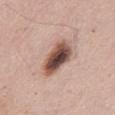Clinical impression: This lesion was catalogued during total-body skin photography and was not selected for biopsy. Context: A 15 mm crop from a total-body photograph taken for skin-cancer surveillance. About 5 mm across. This is a white-light tile. A male subject approximately 55 years of age. The lesion is on the mid back. An algorithmic analysis of the crop reported a footprint of about 12 mm², a shape eccentricity near 0.8, and two-axis asymmetry of about 0.2. The software also gave a classifier nevus-likeness of about 90/100 and a lesion-detection confidence of about 100/100.